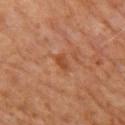{
  "biopsy_status": "not biopsied; imaged during a skin examination",
  "lesion_size": {
    "long_diameter_mm_approx": 2.5
  },
  "patient": {
    "sex": "male",
    "age_approx": 60
  },
  "automated_metrics": {
    "area_mm2_approx": 3.0,
    "shape_asymmetry": 0.3,
    "cielab_L": 44,
    "cielab_a": 25,
    "cielab_b": 34,
    "vs_skin_darker_L": 7.0,
    "vs_skin_contrast_norm": 6.5,
    "border_irregularity_0_10": 2.5,
    "color_variation_0_10": 1.5,
    "peripheral_color_asymmetry": 0.5
  },
  "image": {
    "source": "total-body photography crop",
    "field_of_view_mm": 15
  },
  "site": "left thigh",
  "lighting": "cross-polarized"
}